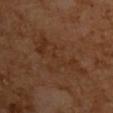No biopsy was performed on this lesion — it was imaged during a full skin examination and was not determined to be concerning. Captured under cross-polarized illumination. Approximately 8 mm at its widest. Cropped from a total-body skin-imaging series; the visible field is about 15 mm. The lesion is on the upper back. A male subject aged 58–62.